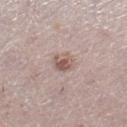biopsy status: no biopsy performed (imaged during a skin exam) | anatomic site: the leg | patient: female, aged 48–52 | image: 15 mm crop, total-body photography | TBP lesion metrics: about 10 CIELAB-L* units darker than the surrounding skin and a lesion-to-skin contrast of about 7.5 (normalized; higher = more distinct).